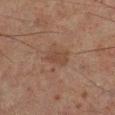Findings:
- notes: no biopsy performed (imaged during a skin exam)
- site: the leg
- image source: 15 mm crop, total-body photography
- patient: male, aged approximately 60